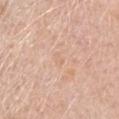Case summary:
– follow-up: imaged on a skin check; not biopsied
– TBP lesion metrics: an eccentricity of roughly 0.6
– patient: female, aged 48–52
– lesion diameter: about 1.5 mm
– lighting: white-light
– location: the head or neck
– image source: ~15 mm tile from a whole-body skin photo